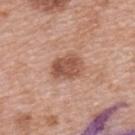Recorded during total-body skin imaging; not selected for excision or biopsy. A 15 mm close-up extracted from a 3D total-body photography capture. A female patient aged approximately 60. The lesion is located on the upper back. The tile uses white-light illumination.